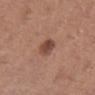Part of a total-body skin-imaging series; this lesion was reviewed on a skin check and was not flagged for biopsy. Automated tile analysis of the lesion measured an average lesion color of about L≈46 a*≈22 b*≈26 (CIELAB), about 12 CIELAB-L* units darker than the surrounding skin, and a normalized lesion–skin contrast near 8.5. And it measured a border-irregularity rating of about 2/10 and a within-lesion color-variation index near 3/10. It also reported a classifier nevus-likeness of about 90/100. Approximately 3 mm at its widest. Captured under white-light illumination. The patient is a female aged around 45. This image is a 15 mm lesion crop taken from a total-body photograph. On the left lower leg.